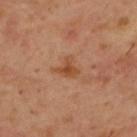Clinical impression:
The lesion was photographed on a routine skin check and not biopsied; there is no pathology result.
Clinical summary:
An algorithmic analysis of the crop reported a lesion color around L≈46 a*≈23 b*≈35 in CIELAB and roughly 8 lightness units darker than nearby skin. And it measured border irregularity of about 5 on a 0–10 scale and internal color variation of about 2.5 on a 0–10 scale. From the back. A male subject roughly 55 years of age. Approximately 2.5 mm at its widest. A roughly 15 mm field-of-view crop from a total-body skin photograph.